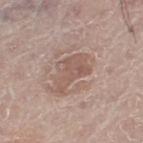The lesion was tiled from a total-body skin photograph and was not biopsied. The patient is a male aged around 70. Located on the right leg. A 15 mm close-up extracted from a 3D total-body photography capture.